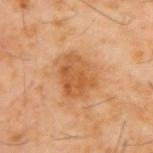Q: Was this lesion biopsied?
A: no biopsy performed (imaged during a skin exam)
Q: What are the patient's age and sex?
A: male, about 60 years old
Q: Where on the body is the lesion?
A: the upper back
Q: How large is the lesion?
A: ~5 mm (longest diameter)
Q: How was the tile lit?
A: cross-polarized
Q: How was this image acquired?
A: ~15 mm tile from a whole-body skin photo
Q: Automated lesion metrics?
A: a footprint of about 16 mm², an outline eccentricity of about 0.55 (0 = round, 1 = elongated), and a symmetry-axis asymmetry near 0.25; border irregularity of about 2.5 on a 0–10 scale and a color-variation rating of about 4.5/10